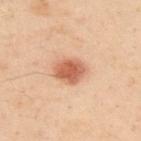Impression:
This lesion was catalogued during total-body skin photography and was not selected for biopsy.
Clinical summary:
A close-up tile cropped from a whole-body skin photograph, about 15 mm across. Approximately 3.5 mm at its widest. The lesion is located on the upper back. The patient is a male about 50 years old. Captured under cross-polarized illumination.